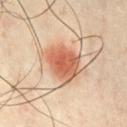Captured during whole-body skin photography for melanoma surveillance; the lesion was not biopsied. About 5 mm across. A roughly 15 mm field-of-view crop from a total-body skin photograph. The subject is a male aged approximately 50. From the chest. Imaged with cross-polarized lighting.